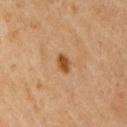Assessment:
Recorded during total-body skin imaging; not selected for excision or biopsy.
Image and clinical context:
A 15 mm crop from a total-body photograph taken for skin-cancer surveillance. Captured under cross-polarized illumination. The subject is a female about 70 years old. Measured at roughly 2.5 mm in maximum diameter. Located on the right upper arm.